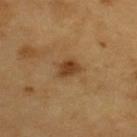biopsy status — catalogued during a skin exam; not biopsied
tile lighting — cross-polarized illumination
subject — male, in their 60s
acquisition — ~15 mm tile from a whole-body skin photo
body site — the upper back